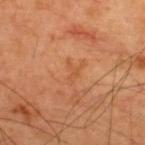Clinical impression: Recorded during total-body skin imaging; not selected for excision or biopsy. Context: The lesion is on the back. A 15 mm close-up extracted from a 3D total-body photography capture. Captured under cross-polarized illumination. A male subject, aged 58 to 62.